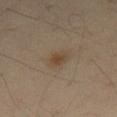{
  "biopsy_status": "not biopsied; imaged during a skin examination",
  "site": "front of the torso",
  "image": {
    "source": "total-body photography crop",
    "field_of_view_mm": 15
  },
  "patient": {
    "sex": "male",
    "age_approx": 55
  },
  "lighting": "cross-polarized",
  "lesion_size": {
    "long_diameter_mm_approx": 3.0
  },
  "automated_metrics": {
    "cielab_L": 44,
    "cielab_a": 14,
    "cielab_b": 28,
    "vs_skin_darker_L": 7.0,
    "vs_skin_contrast_norm": 6.5,
    "nevus_likeness_0_100": 70,
    "lesion_detection_confidence_0_100": 100
  }
}